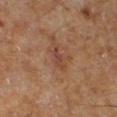| field | value |
|---|---|
| biopsy status | no biopsy performed (imaged during a skin exam) |
| patient | male, aged around 60 |
| imaging modality | ~15 mm tile from a whole-body skin photo |
| anatomic site | the right lower leg |